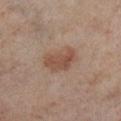Assessment:
The lesion was tiled from a total-body skin photograph and was not biopsied.
Acquisition and patient details:
A female subject, in their 50s. A close-up tile cropped from a whole-body skin photograph, about 15 mm across. Located on the right lower leg. Imaged with cross-polarized lighting. Approximately 4 mm at its widest.